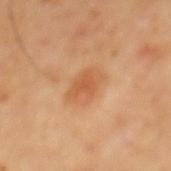Impression:
Imaged during a routine full-body skin examination; the lesion was not biopsied and no histopathology is available.
Context:
From the mid back. A male patient aged around 65. A 15 mm close-up extracted from a 3D total-body photography capture. This is a cross-polarized tile. The recorded lesion diameter is about 3.5 mm. Automated tile analysis of the lesion measured a lesion color around L≈57 a*≈25 b*≈40 in CIELAB. And it measured an automated nevus-likeness rating near 85 out of 100 and lesion-presence confidence of about 100/100.